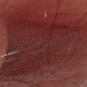{
  "biopsy_status": "not biopsied; imaged during a skin examination",
  "patient": {
    "sex": "female",
    "age_approx": 55
  },
  "site": "head or neck",
  "lighting": "white-light",
  "image": {
    "source": "total-body photography crop",
    "field_of_view_mm": 15
  },
  "lesion_size": {
    "long_diameter_mm_approx": 21.5
  },
  "automated_metrics": {
    "vs_skin_darker_L": 11.0,
    "vs_skin_contrast_norm": 9.5,
    "border_irregularity_0_10": 6.0
  }
}